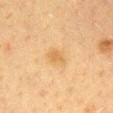Background:
This image is a 15 mm lesion crop taken from a total-body photograph. The lesion's longest dimension is about 2.5 mm. The total-body-photography lesion software estimated an average lesion color of about L≈54 a*≈16 b*≈37 (CIELAB), about 7 CIELAB-L* units darker than the surrounding skin, and a normalized border contrast of about 5.5. The software also gave a classifier nevus-likeness of about 25/100 and a lesion-detection confidence of about 100/100. Located on the chest. A female patient aged 38 to 42.